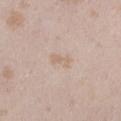| key | value |
|---|---|
| follow-up | catalogued during a skin exam; not biopsied |
| TBP lesion metrics | a border-irregularity index near 3/10, internal color variation of about 1.5 on a 0–10 scale, and peripheral color asymmetry of about 0.5; a classifier nevus-likeness of about 0/100 and a lesion-detection confidence of about 100/100 |
| body site | the leg |
| image source | total-body-photography crop, ~15 mm field of view |
| lighting | white-light illumination |
| patient | female, aged approximately 25 |
| lesion diameter | about 3 mm |